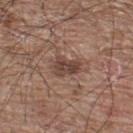notes=catalogued during a skin exam; not biopsied | anatomic site=the back | patient=male, roughly 65 years of age | tile lighting=white-light | automated metrics=a border-irregularity rating of about 3.5/10, a color-variation rating of about 4/10, and radial color variation of about 1.5 | acquisition=15 mm crop, total-body photography | diameter=about 3.5 mm.